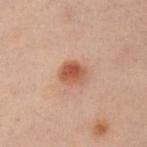biopsy status: no biopsy performed (imaged during a skin exam) | body site: the left arm | illumination: cross-polarized illumination | subject: male, aged 48–52 | lesion diameter: about 3 mm | image source: ~15 mm crop, total-body skin-cancer survey.